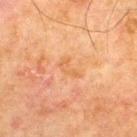This lesion was catalogued during total-body skin photography and was not selected for biopsy. A 15 mm close-up extracted from a 3D total-body photography capture. About 3 mm across. Located on the upper back. A male patient, aged around 70. The lesion-visualizer software estimated a lesion color around L≈54 a*≈21 b*≈36 in CIELAB and about 5 CIELAB-L* units darker than the surrounding skin. And it measured border irregularity of about 5 on a 0–10 scale, internal color variation of about 1.5 on a 0–10 scale, and a peripheral color-asymmetry measure near 0.5.